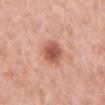Clinical impression: Recorded during total-body skin imaging; not selected for excision or biopsy. Acquisition and patient details: A female patient, aged around 55. The lesion's longest dimension is about 3.5 mm. The tile uses white-light illumination. Automated image analysis of the tile measured a mean CIELAB color near L≈56 a*≈27 b*≈30, roughly 13 lightness units darker than nearby skin, and a normalized border contrast of about 8.5. And it measured a border-irregularity rating of about 1.5/10, internal color variation of about 4 on a 0–10 scale, and radial color variation of about 1. The analysis additionally found an automated nevus-likeness rating near 95 out of 100. The lesion is on the right lower leg. A close-up tile cropped from a whole-body skin photograph, about 15 mm across.